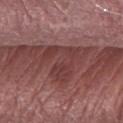Part of a total-body skin-imaging series; this lesion was reviewed on a skin check and was not flagged for biopsy. A 15 mm close-up extracted from a 3D total-body photography capture. A male subject aged 63 to 67. On the right forearm.